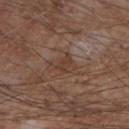Clinical impression: The lesion was photographed on a routine skin check and not biopsied; there is no pathology result.